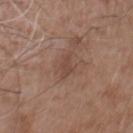The lesion was tiled from a total-body skin photograph and was not biopsied.
A male patient about 75 years old.
Automated tile analysis of the lesion measured a lesion color around L≈45 a*≈19 b*≈25 in CIELAB, roughly 7 lightness units darker than nearby skin, and a normalized border contrast of about 5.5.
Imaged with white-light lighting.
Longest diameter approximately 3 mm.
The lesion is on the chest.
A 15 mm close-up extracted from a 3D total-body photography capture.